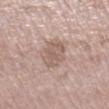Clinical impression:
Recorded during total-body skin imaging; not selected for excision or biopsy.